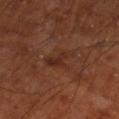notes — imaged on a skin check; not biopsied
location — the right lower leg
diameter — about 4 mm
illumination — cross-polarized illumination
image-analysis metrics — a lesion area of about 6 mm², an outline eccentricity of about 0.75 (0 = round, 1 = elongated), and two-axis asymmetry of about 0.4; a nevus-likeness score of about 0/100 and a lesion-detection confidence of about 100/100
image — 15 mm crop, total-body photography
patient — male, about 70 years old